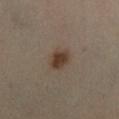workup: imaged on a skin check; not biopsied
patient: female, aged 38–42
automated metrics: a mean CIELAB color near L≈38 a*≈15 b*≈25, a lesion–skin lightness drop of about 11, and a lesion-to-skin contrast of about 9.5 (normalized; higher = more distinct); a border-irregularity rating of about 1.5/10 and radial color variation of about 0.5; lesion-presence confidence of about 100/100
lighting: cross-polarized
lesion size: ≈2.5 mm
site: the left leg
image source: ~15 mm tile from a whole-body skin photo